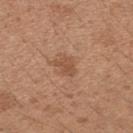biopsy status: no biopsy performed (imaged during a skin exam) | subject: female, approximately 30 years of age | size: ~3 mm (longest diameter) | image: ~15 mm tile from a whole-body skin photo | body site: the right forearm | lighting: white-light.